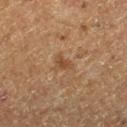This lesion was catalogued during total-body skin photography and was not selected for biopsy. A male subject, roughly 75 years of age. From the left thigh. The lesion-visualizer software estimated border irregularity of about 3.5 on a 0–10 scale, internal color variation of about 1.5 on a 0–10 scale, and a peripheral color-asymmetry measure near 0.5. The analysis additionally found lesion-presence confidence of about 100/100. This is a cross-polarized tile. Longest diameter approximately 2.5 mm. A close-up tile cropped from a whole-body skin photograph, about 15 mm across.